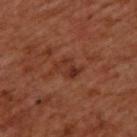Imaged during a routine full-body skin examination; the lesion was not biopsied and no histopathology is available.
The total-body-photography lesion software estimated an area of roughly 4 mm², a shape eccentricity near 0.75, and a shape-asymmetry score of about 0.5 (0 = symmetric). It also reported border irregularity of about 6.5 on a 0–10 scale, a within-lesion color-variation index near 1/10, and peripheral color asymmetry of about 0.5. The software also gave a lesion-detection confidence of about 100/100.
A region of skin cropped from a whole-body photographic capture, roughly 15 mm wide.
On the upper back.
The patient is a male roughly 50 years of age.
About 3 mm across.